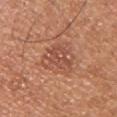Q: Is there a histopathology result?
A: catalogued during a skin exam; not biopsied
Q: How was this image acquired?
A: ~15 mm tile from a whole-body skin photo
Q: How was the tile lit?
A: white-light
Q: What are the patient's age and sex?
A: male, aged around 30
Q: How large is the lesion?
A: about 5 mm
Q: Where on the body is the lesion?
A: the right upper arm
Q: Automated lesion metrics?
A: a footprint of about 11 mm² and an eccentricity of roughly 0.8; an average lesion color of about L≈51 a*≈25 b*≈31 (CIELAB); a border-irregularity rating of about 4.5/10, a within-lesion color-variation index near 5/10, and a peripheral color-asymmetry measure near 2; a classifier nevus-likeness of about 5/100